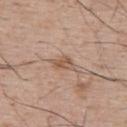{"biopsy_status": "not biopsied; imaged during a skin examination", "lighting": "white-light", "lesion_size": {"long_diameter_mm_approx": 3.0}, "image": {"source": "total-body photography crop", "field_of_view_mm": 15}, "automated_metrics": {"eccentricity": 0.85, "vs_skin_darker_L": 9.0, "vs_skin_contrast_norm": 6.5, "border_irregularity_0_10": 3.0, "color_variation_0_10": 3.0, "peripheral_color_asymmetry": 1.0}, "site": "upper back", "patient": {"sex": "male", "age_approx": 65}}